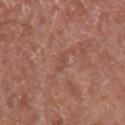Assessment:
Captured during whole-body skin photography for melanoma surveillance; the lesion was not biopsied.
Image and clinical context:
This is a white-light tile. The total-body-photography lesion software estimated an automated nevus-likeness rating near 0 out of 100 and a lesion-detection confidence of about 65/100. From the left upper arm. A male subject, aged 78–82. A close-up tile cropped from a whole-body skin photograph, about 15 mm across. About 2.5 mm across.